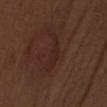Q: What lighting was used for the tile?
A: white-light
Q: How was this image acquired?
A: ~15 mm crop, total-body skin-cancer survey
Q: Where on the body is the lesion?
A: the head or neck
Q: Patient demographics?
A: female, aged 48–52
Q: How large is the lesion?
A: about 5.5 mm